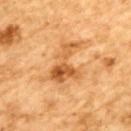| field | value |
|---|---|
| notes | no biopsy performed (imaged during a skin exam) |
| automated metrics | a footprint of about 11 mm², an eccentricity of roughly 0.85, and a shape-asymmetry score of about 0.7 (0 = symmetric) |
| patient | male, approximately 85 years of age or older |
| imaging modality | ~15 mm crop, total-body skin-cancer survey |
| diameter | about 5.5 mm |
| illumination | cross-polarized |
| location | the upper back |